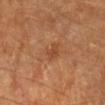anatomic site — the left forearm
illumination — cross-polarized illumination
subject — female, aged around 60
acquisition — 15 mm crop, total-body photography
lesion diameter — ≈2.5 mm
TBP lesion metrics — an area of roughly 3 mm², an eccentricity of roughly 0.85, and a shape-asymmetry score of about 0.25 (0 = symmetric); an average lesion color of about L≈47 a*≈25 b*≈36 (CIELAB) and a lesion–skin lightness drop of about 8; border irregularity of about 2.5 on a 0–10 scale, a color-variation rating of about 1/10, and a peripheral color-asymmetry measure near 0.5; an automated nevus-likeness rating near 0 out of 100